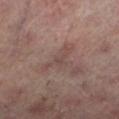Case summary:
* biopsy status: no biopsy performed (imaged during a skin exam)
* size: ~4 mm (longest diameter)
* location: the left lower leg
* image: ~15 mm tile from a whole-body skin photo
* automated lesion analysis: an area of roughly 5.5 mm², an outline eccentricity of about 0.8 (0 = round, 1 = elongated), and a shape-asymmetry score of about 0.6 (0 = symmetric); a border-irregularity index near 8/10
* patient: male, in their mid- to late 60s